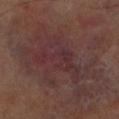A 15 mm close-up extracted from a 3D total-body photography capture. From the left lower leg. The recorded lesion diameter is about 6.5 mm. Automated tile analysis of the lesion measured an area of roughly 18 mm² and two-axis asymmetry of about 0.55. Captured under cross-polarized illumination.